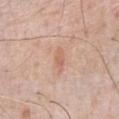– follow-up — no biopsy performed (imaged during a skin exam)
– lighting — white-light illumination
– imaging modality — ~15 mm crop, total-body skin-cancer survey
– site — the chest
– subject — male, about 80 years old
– lesion size — ≈3 mm